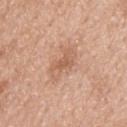follow-up = catalogued during a skin exam; not biopsied
subject = male, about 50 years old
automated lesion analysis = a lesion area of about 7 mm², a shape eccentricity near 0.85, and a symmetry-axis asymmetry near 0.35; an average lesion color of about L≈60 a*≈21 b*≈32 (CIELAB), a lesion–skin lightness drop of about 8, and a normalized border contrast of about 5.5; border irregularity of about 4.5 on a 0–10 scale, internal color variation of about 3 on a 0–10 scale, and peripheral color asymmetry of about 1
body site = the upper back
image = ~15 mm crop, total-body skin-cancer survey
lesion diameter = about 4 mm